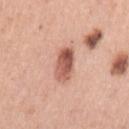<record>
  <biopsy_status>not biopsied; imaged during a skin examination</biopsy_status>
  <lesion_size>
    <long_diameter_mm_approx>4.0</long_diameter_mm_approx>
  </lesion_size>
  <image>
    <source>total-body photography crop</source>
    <field_of_view_mm>15</field_of_view_mm>
  </image>
  <automated_metrics>
    <nevus_likeness_0_100>95</nevus_likeness_0_100>
    <lesion_detection_confidence_0_100>100</lesion_detection_confidence_0_100>
  </automated_metrics>
  <site>left upper arm</site>
  <lighting>white-light</lighting>
  <patient>
    <sex>female</sex>
    <age_approx>60</age_approx>
  </patient>
</record>